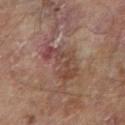Clinical impression:
This lesion was catalogued during total-body skin photography and was not selected for biopsy.
Acquisition and patient details:
The subject is a male in their mid-80s or older. On the right forearm. A 15 mm close-up tile from a total-body photography series done for melanoma screening. Imaged with cross-polarized lighting. Longest diameter approximately 6 mm. The total-body-photography lesion software estimated a shape eccentricity near 0.9 and two-axis asymmetry of about 0.5. And it measured a mean CIELAB color near L≈42 a*≈19 b*≈23, roughly 8 lightness units darker than nearby skin, and a normalized border contrast of about 6.5. The software also gave a border-irregularity rating of about 7/10, a color-variation rating of about 4/10, and peripheral color asymmetry of about 1. The software also gave an automated nevus-likeness rating near 0 out of 100 and lesion-presence confidence of about 90/100.